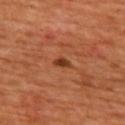About 2 mm across. The patient is female. The tile uses cross-polarized illumination. Automated tile analysis of the lesion measured about 11 CIELAB-L* units darker than the surrounding skin and a normalized border contrast of about 9. The analysis additionally found a border-irregularity rating of about 3/10, a color-variation rating of about 1.5/10, and a peripheral color-asymmetry measure near 0.5. The analysis additionally found a classifier nevus-likeness of about 80/100 and a detector confidence of about 100 out of 100 that the crop contains a lesion. Located on the upper back. Cropped from a total-body skin-imaging series; the visible field is about 15 mm.